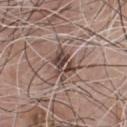workup = catalogued during a skin exam; not biopsied
automated metrics = a footprint of about 7.5 mm², an eccentricity of roughly 0.7, and a symmetry-axis asymmetry near 0.25
imaging modality = ~15 mm tile from a whole-body skin photo
lighting = white-light
patient = male, in their mid-70s
size = ≈3.5 mm
location = the front of the torso